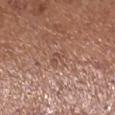Clinical summary: A 15 mm close-up tile from a total-body photography series done for melanoma screening. The patient is a female aged 53–57. Longest diameter approximately 2.5 mm. The lesion is on the right lower leg.